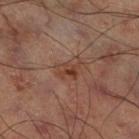Q: How was the tile lit?
A: cross-polarized illumination
Q: What is the imaging modality?
A: ~15 mm tile from a whole-body skin photo
Q: Where on the body is the lesion?
A: the right thigh